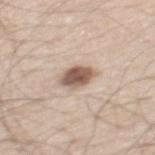Q: What kind of image is this?
A: ~15 mm crop, total-body skin-cancer survey
Q: Where on the body is the lesion?
A: the mid back
Q: Automated lesion metrics?
A: a footprint of about 7.5 mm² and an outline eccentricity of about 0.7 (0 = round, 1 = elongated)
Q: Patient demographics?
A: male, roughly 60 years of age
Q: What lighting was used for the tile?
A: white-light illumination
Q: How large is the lesion?
A: about 3.5 mm